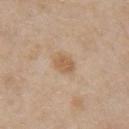Recorded during total-body skin imaging; not selected for excision or biopsy.
The tile uses white-light illumination.
Approximately 3 mm at its widest.
A female subject aged 43 to 47.
A roughly 15 mm field-of-view crop from a total-body skin photograph.
On the chest.
The total-body-photography lesion software estimated a footprint of about 5 mm², an outline eccentricity of about 0.65 (0 = round, 1 = elongated), and two-axis asymmetry of about 0.15. The analysis additionally found border irregularity of about 1.5 on a 0–10 scale, a color-variation rating of about 1.5/10, and a peripheral color-asymmetry measure near 0.5. The analysis additionally found a nevus-likeness score of about 35/100 and a detector confidence of about 100 out of 100 that the crop contains a lesion.